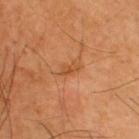{
  "biopsy_status": "not biopsied; imaged during a skin examination",
  "patient": {
    "sex": "male",
    "age_approx": 45
  },
  "site": "back",
  "image": {
    "source": "total-body photography crop",
    "field_of_view_mm": 15
  }
}